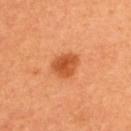Part of a total-body skin-imaging series; this lesion was reviewed on a skin check and was not flagged for biopsy. A female subject aged 48 to 52. Approximately 3.5 mm at its widest. A roughly 15 mm field-of-view crop from a total-body skin photograph.